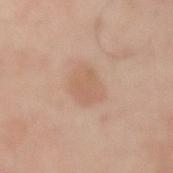Captured during whole-body skin photography for melanoma surveillance; the lesion was not biopsied.